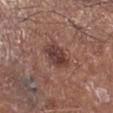follow-up: imaged on a skin check; not biopsied
imaging modality: 15 mm crop, total-body photography
anatomic site: the right lower leg
automated lesion analysis: an average lesion color of about L≈40 a*≈20 b*≈22 (CIELAB), roughly 10 lightness units darker than nearby skin, and a normalized lesion–skin contrast near 9; border irregularity of about 1.5 on a 0–10 scale and a peripheral color-asymmetry measure near 1.5; an automated nevus-likeness rating near 80 out of 100 and a detector confidence of about 100 out of 100 that the crop contains a lesion
subject: male, aged approximately 70
tile lighting: white-light